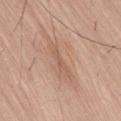Captured during whole-body skin photography for melanoma surveillance; the lesion was not biopsied.